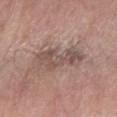Part of a total-body skin-imaging series; this lesion was reviewed on a skin check and was not flagged for biopsy. From the right forearm. A male patient, aged 58–62. Cropped from a total-body skin-imaging series; the visible field is about 15 mm.